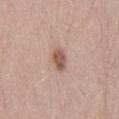Automated image analysis of the tile measured an area of roughly 4.5 mm² and an outline eccentricity of about 0.8 (0 = round, 1 = elongated). And it measured a border-irregularity index near 2/10, internal color variation of about 3 on a 0–10 scale, and a peripheral color-asymmetry measure near 1. It also reported a classifier nevus-likeness of about 95/100 and a lesion-detection confidence of about 100/100. A male patient about 45 years old. This is a white-light tile. The lesion is located on the right thigh. Approximately 3 mm at its widest. A region of skin cropped from a whole-body photographic capture, roughly 15 mm wide.